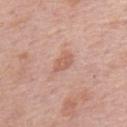Imaged during a routine full-body skin examination; the lesion was not biopsied and no histopathology is available. The recorded lesion diameter is about 3 mm. On the upper back. The tile uses white-light illumination. A region of skin cropped from a whole-body photographic capture, roughly 15 mm wide. The subject is a male aged 73–77.